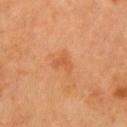<tbp_lesion>
  <biopsy_status>not biopsied; imaged during a skin examination</biopsy_status>
</tbp_lesion>